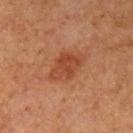notes — catalogued during a skin exam; not biopsied
lesion diameter — ≈4.5 mm
illumination — cross-polarized
acquisition — total-body-photography crop, ~15 mm field of view
subject — female, about 60 years old
TBP lesion metrics — a lesion color around L≈40 a*≈24 b*≈31 in CIELAB, roughly 8 lightness units darker than nearby skin, and a normalized lesion–skin contrast near 6.5; a classifier nevus-likeness of about 80/100
site — the arm